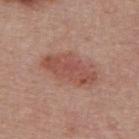Clinical impression: Part of a total-body skin-imaging series; this lesion was reviewed on a skin check and was not flagged for biopsy. Image and clinical context: Cropped from a total-body skin-imaging series; the visible field is about 15 mm. The lesion is on the upper back. The subject is a female aged approximately 50. Automated image analysis of the tile measured about 9 CIELAB-L* units darker than the surrounding skin and a normalized lesion–skin contrast near 7. And it measured a border-irregularity index near 3/10 and a within-lesion color-variation index near 3.5/10. The software also gave an automated nevus-likeness rating near 95 out of 100 and a detector confidence of about 100 out of 100 that the crop contains a lesion. Longest diameter approximately 6 mm.